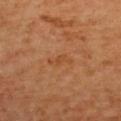Imaged during a routine full-body skin examination; the lesion was not biopsied and no histopathology is available.
The patient is female.
Cropped from a whole-body photographic skin survey; the tile spans about 15 mm.
On the upper back.